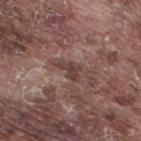The lesion was photographed on a routine skin check and not biopsied; there is no pathology result.
Captured under white-light illumination.
A roughly 15 mm field-of-view crop from a total-body skin photograph.
On the right thigh.
The lesion's longest dimension is about 3 mm.
A male subject, aged 73 to 77.